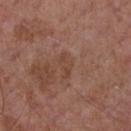patient = male, in their mid- to late 50s | image source = total-body-photography crop, ~15 mm field of view | location = the chest.